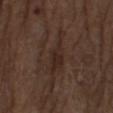Q: Is there a histopathology result?
A: imaged on a skin check; not biopsied
Q: What is the imaging modality?
A: ~15 mm crop, total-body skin-cancer survey
Q: Lesion location?
A: the left thigh
Q: What lighting was used for the tile?
A: white-light illumination
Q: Lesion size?
A: ~6.5 mm (longest diameter)
Q: Patient demographics?
A: male, about 75 years old
Q: What did automated image analysis measure?
A: an area of roughly 9 mm², a shape eccentricity near 0.95, and a shape-asymmetry score of about 0.45 (0 = symmetric); a border-irregularity rating of about 6.5/10, a within-lesion color-variation index near 2.5/10, and peripheral color asymmetry of about 1; a nevus-likeness score of about 0/100 and a detector confidence of about 55 out of 100 that the crop contains a lesion